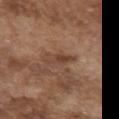image source: 15 mm crop, total-body photography; anatomic site: the chest; illumination: white-light illumination; subject: male, aged 73–77.